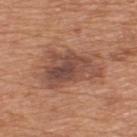| key | value |
|---|---|
| follow-up | total-body-photography surveillance lesion; no biopsy |
| patient | male, approximately 65 years of age |
| lighting | white-light |
| site | the upper back |
| image-analysis metrics | an area of roughly 23 mm², an outline eccentricity of about 0.85 (0 = round, 1 = elongated), and a symmetry-axis asymmetry near 0.3; an average lesion color of about L≈48 a*≈22 b*≈28 (CIELAB), roughly 11 lightness units darker than nearby skin, and a lesion-to-skin contrast of about 8.5 (normalized; higher = more distinct); a border-irregularity index near 4/10, a within-lesion color-variation index near 6/10, and radial color variation of about 2; a classifier nevus-likeness of about 35/100 |
| image source | 15 mm crop, total-body photography |
| lesion size | about 7.5 mm |